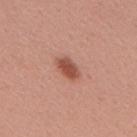Captured during whole-body skin photography for melanoma surveillance; the lesion was not biopsied. Automated image analysis of the tile measured a shape-asymmetry score of about 0.2 (0 = symmetric). The software also gave a lesion-detection confidence of about 100/100. From the upper back. A female patient in their mid- to late 40s. This image is a 15 mm lesion crop taken from a total-body photograph. About 3 mm across. Imaged with white-light lighting.